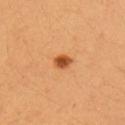Clinical impression:
This lesion was catalogued during total-body skin photography and was not selected for biopsy.
Context:
Cropped from a total-body skin-imaging series; the visible field is about 15 mm. The subject is a female approximately 40 years of age. From the right upper arm.